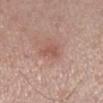Case summary:
• biopsy status — total-body-photography surveillance lesion; no biopsy
• site — the right lower leg
• automated lesion analysis — a footprint of about 3.5 mm² and an eccentricity of roughly 0.7; a border-irregularity index near 4/10 and a color-variation rating of about 1/10; a lesion-detection confidence of about 100/100
• lighting — white-light
• subject — male, aged around 55
• imaging modality — ~15 mm tile from a whole-body skin photo